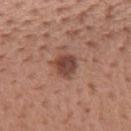| field | value |
|---|---|
| anatomic site | the mid back |
| subject | male, approximately 55 years of age |
| illumination | white-light illumination |
| image | total-body-photography crop, ~15 mm field of view |
| lesion diameter | ~3 mm (longest diameter) |